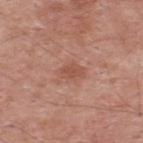notes: no biopsy performed (imaged during a skin exam)
location: the upper back
subject: male, aged 53 to 57
image: ~15 mm crop, total-body skin-cancer survey